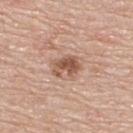{"biopsy_status": "not biopsied; imaged during a skin examination", "image": {"source": "total-body photography crop", "field_of_view_mm": 15}, "patient": {"sex": "male", "age_approx": 70}, "automated_metrics": {"border_irregularity_0_10": 3.5, "color_variation_0_10": 4.0, "peripheral_color_asymmetry": 1.5}, "site": "upper back", "lighting": "white-light", "lesion_size": {"long_diameter_mm_approx": 3.5}}